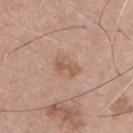Background: From the front of the torso. The recorded lesion diameter is about 3 mm. Captured under white-light illumination. A close-up tile cropped from a whole-body skin photograph, about 15 mm across. The subject is a male aged 73 to 77.